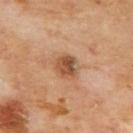Clinical impression: Captured during whole-body skin photography for melanoma surveillance; the lesion was not biopsied. Clinical summary: The subject is a male in their 70s. Located on the back. A 15 mm close-up extracted from a 3D total-body photography capture. The tile uses cross-polarized illumination. Longest diameter approximately 3 mm.